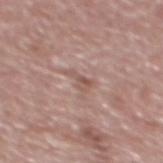Impression:
Imaged during a routine full-body skin examination; the lesion was not biopsied and no histopathology is available.
Acquisition and patient details:
Captured under white-light illumination. A 15 mm crop from a total-body photograph taken for skin-cancer surveillance. The lesion is on the mid back. The total-body-photography lesion software estimated a footprint of about 3 mm², an outline eccentricity of about 0.8 (0 = round, 1 = elongated), and a symmetry-axis asymmetry near 0.55. The analysis additionally found a border-irregularity rating of about 6/10, a within-lesion color-variation index near 1.5/10, and a peripheral color-asymmetry measure near 0.5. The lesion's longest dimension is about 2.5 mm. The patient is a male aged 68 to 72.